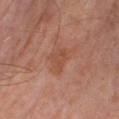Imaged during a routine full-body skin examination; the lesion was not biopsied and no histopathology is available. Cropped from a whole-body photographic skin survey; the tile spans about 15 mm. This is a cross-polarized tile. Longest diameter approximately 3 mm. A male subject, in their mid- to late 60s. The lesion is on the right lower leg.